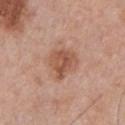Impression: No biopsy was performed on this lesion — it was imaged during a full skin examination and was not determined to be concerning. Image and clinical context: The subject is a male about 60 years old. From the chest. A 15 mm crop from a total-body photograph taken for skin-cancer surveillance. About 4 mm across.